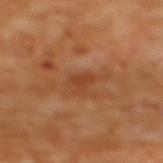The lesion was tiled from a total-body skin photograph and was not biopsied.
This is a cross-polarized tile.
The recorded lesion diameter is about 2.5 mm.
The lesion-visualizer software estimated a lesion area of about 3.5 mm², an outline eccentricity of about 0.8 (0 = round, 1 = elongated), and a symmetry-axis asymmetry near 0.5. It also reported a mean CIELAB color near L≈44 a*≈25 b*≈38, about 7 CIELAB-L* units darker than the surrounding skin, and a lesion-to-skin contrast of about 6 (normalized; higher = more distinct). And it measured a border-irregularity rating of about 5/10, a color-variation rating of about 0.5/10, and a peripheral color-asymmetry measure near 0.5.
A 15 mm crop from a total-body photograph taken for skin-cancer surveillance.
On the upper back.
A female subject in their mid- to late 50s.